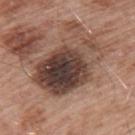This lesion was catalogued during total-body skin photography and was not selected for biopsy. A male subject in their mid-50s. A lesion tile, about 15 mm wide, cut from a 3D total-body photograph. This is a white-light tile. Located on the back.